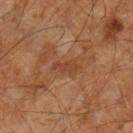site: the right lower leg | lesion size: ≈3.5 mm | subject: male, about 60 years old | imaging modality: ~15 mm tile from a whole-body skin photo | tile lighting: cross-polarized illumination.